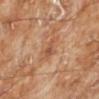Cropped from a whole-body photographic skin survey; the tile spans about 15 mm.
Located on the left lower leg.
The subject is a male aged around 70.
This is a cross-polarized tile.
Longest diameter approximately 2.5 mm.